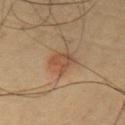The lesion was tiled from a total-body skin photograph and was not biopsied.